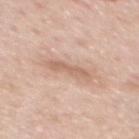Findings:
- follow-up · total-body-photography surveillance lesion; no biopsy
- location · the back
- tile lighting · white-light
- lesion diameter · ≈4.5 mm
- subject · male, approximately 60 years of age
- acquisition · 15 mm crop, total-body photography